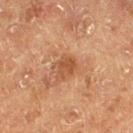Recorded during total-body skin imaging; not selected for excision or biopsy. The lesion is on the left lower leg. The recorded lesion diameter is about 2.5 mm. Captured under cross-polarized illumination. A lesion tile, about 15 mm wide, cut from a 3D total-body photograph. A male subject, aged around 75.